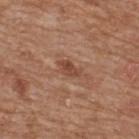Assessment: The lesion was tiled from a total-body skin photograph and was not biopsied.